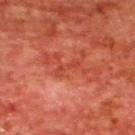No biopsy was performed on this lesion — it was imaged during a full skin examination and was not determined to be concerning. A lesion tile, about 15 mm wide, cut from a 3D total-body photograph. The patient is a male roughly 65 years of age. On the upper back.